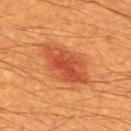follow-up: no biopsy performed (imaged during a skin exam); site: the mid back; tile lighting: cross-polarized illumination; image source: ~15 mm tile from a whole-body skin photo; patient: male, aged approximately 60.